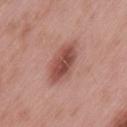<record>
<biopsy_status>not biopsied; imaged during a skin examination</biopsy_status>
<image>
  <source>total-body photography crop</source>
  <field_of_view_mm>15</field_of_view_mm>
</image>
<patient>
  <sex>male</sex>
  <age_approx>55</age_approx>
</patient>
<lighting>white-light</lighting>
<lesion_size>
  <long_diameter_mm_approx>5.5</long_diameter_mm_approx>
</lesion_size>
<site>lower back</site>
</record>